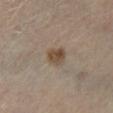Part of a total-body skin-imaging series; this lesion was reviewed on a skin check and was not flagged for biopsy. The lesion is located on the left lower leg. This is a cross-polarized tile. Cropped from a total-body skin-imaging series; the visible field is about 15 mm. A female patient, aged 58–62. The lesion's longest dimension is about 2.5 mm. The lesion-visualizer software estimated an eccentricity of roughly 0.5. The software also gave a mean CIELAB color near L≈46 a*≈13 b*≈27, about 10 CIELAB-L* units darker than the surrounding skin, and a normalized border contrast of about 8.5. It also reported a peripheral color-asymmetry measure near 1.5.